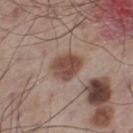Captured during whole-body skin photography for melanoma surveillance; the lesion was not biopsied. An algorithmic analysis of the crop reported a symmetry-axis asymmetry near 0.2. The software also gave a mean CIELAB color near L≈46 a*≈19 b*≈24, about 12 CIELAB-L* units darker than the surrounding skin, and a lesion-to-skin contrast of about 9.5 (normalized; higher = more distinct). A male patient aged approximately 60. The tile uses white-light illumination. The lesion's longest dimension is about 3.5 mm. Located on the left thigh. Cropped from a whole-body photographic skin survey; the tile spans about 15 mm.